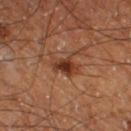Clinical impression:
The lesion was tiled from a total-body skin photograph and was not biopsied.
Background:
Longest diameter approximately 3.5 mm. Automated tile analysis of the lesion measured a footprint of about 6 mm² and a shape-asymmetry score of about 0.35 (0 = symmetric). And it measured a lesion color around L≈34 a*≈22 b*≈29 in CIELAB, a lesion–skin lightness drop of about 10, and a normalized lesion–skin contrast near 9. And it measured border irregularity of about 4 on a 0–10 scale, a color-variation rating of about 5/10, and peripheral color asymmetry of about 1.5. From the right thigh. A male subject aged 58 to 62. A roughly 15 mm field-of-view crop from a total-body skin photograph.